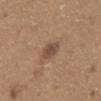Background:
A 15 mm close-up extracted from a 3D total-body photography capture. From the front of the torso. The recorded lesion diameter is about 2.5 mm. Imaged with white-light lighting. A male patient aged 63 to 67.